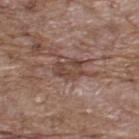This lesion was catalogued during total-body skin photography and was not selected for biopsy.
The recorded lesion diameter is about 3.5 mm.
The lesion is on the upper back.
The tile uses white-light illumination.
A male subject aged approximately 70.
Cropped from a total-body skin-imaging series; the visible field is about 15 mm.